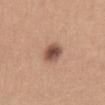Acquisition and patient details: A roughly 15 mm field-of-view crop from a total-body skin photograph. Measured at roughly 3 mm in maximum diameter. This is a white-light tile. A female subject, roughly 30 years of age. Located on the front of the torso.